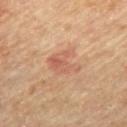This lesion was catalogued during total-body skin photography and was not selected for biopsy. The patient is a male approximately 85 years of age or older. Cropped from a total-body skin-imaging series; the visible field is about 15 mm. Located on the mid back. The lesion's longest dimension is about 4.5 mm. The total-body-photography lesion software estimated a lesion color around L≈58 a*≈23 b*≈31 in CIELAB, a lesion–skin lightness drop of about 8, and a lesion-to-skin contrast of about 5 (normalized; higher = more distinct). The software also gave internal color variation of about 4.5 on a 0–10 scale and peripheral color asymmetry of about 1.5. This is a cross-polarized tile.